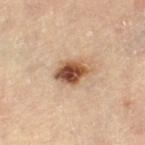Clinical impression: Recorded during total-body skin imaging; not selected for excision or biopsy. Context: A 15 mm close-up tile from a total-body photography series done for melanoma screening. Located on the left thigh. The patient is a male in their mid-80s or older.